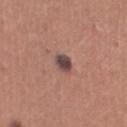Imaged during a routine full-body skin examination; the lesion was not biopsied and no histopathology is available.
This is a white-light tile.
About 2.5 mm across.
Cropped from a total-body skin-imaging series; the visible field is about 15 mm.
From the right thigh.
The lesion-visualizer software estimated a lesion area of about 4.5 mm² and a shape eccentricity near 0.35. And it measured a border-irregularity index near 2/10, a within-lesion color-variation index near 5.5/10, and radial color variation of about 1.5.
A female patient aged 33 to 37.